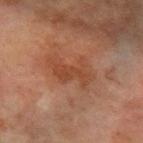Acquisition and patient details: Captured under cross-polarized illumination. Cropped from a whole-body photographic skin survey; the tile spans about 15 mm. A female subject, roughly 55 years of age. The lesion is on the left forearm. Approximately 5.5 mm at its widest.